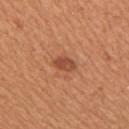This lesion was catalogued during total-body skin photography and was not selected for biopsy. A male patient aged approximately 55. The lesion-visualizer software estimated an area of roughly 4 mm², a shape eccentricity near 0.75, and two-axis asymmetry of about 0.15. It also reported a lesion color around L≈49 a*≈27 b*≈34 in CIELAB, roughly 10 lightness units darker than nearby skin, and a normalized border contrast of about 7.5. And it measured a border-irregularity rating of about 1.5/10 and internal color variation of about 2.5 on a 0–10 scale. And it measured a classifier nevus-likeness of about 85/100 and a lesion-detection confidence of about 100/100. The lesion is on the arm. A roughly 15 mm field-of-view crop from a total-body skin photograph. The tile uses white-light illumination.